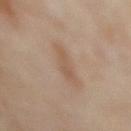Assessment: The lesion was tiled from a total-body skin photograph and was not biopsied. Background: A female subject aged 48–52. Located on the abdomen. A close-up tile cropped from a whole-body skin photograph, about 15 mm across.